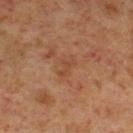Clinical impression: Part of a total-body skin-imaging series; this lesion was reviewed on a skin check and was not flagged for biopsy. Background: The recorded lesion diameter is about 3 mm. Imaged with cross-polarized lighting. A male subject, roughly 60 years of age. Cropped from a total-body skin-imaging series; the visible field is about 15 mm. On the right lower leg. Automated tile analysis of the lesion measured an area of roughly 3.5 mm², an eccentricity of roughly 0.85, and two-axis asymmetry of about 0.45. The software also gave a lesion color around L≈43 a*≈22 b*≈31 in CIELAB, a lesion–skin lightness drop of about 5, and a normalized lesion–skin contrast near 4.5. It also reported an automated nevus-likeness rating near 0 out of 100 and a lesion-detection confidence of about 100/100.